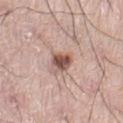notes: imaged on a skin check; not biopsied.